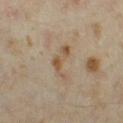notes = catalogued during a skin exam; not biopsied | imaging modality = 15 mm crop, total-body photography | subject = female, in their mid-30s | automated lesion analysis = a footprint of about 5.5 mm² and a symmetry-axis asymmetry near 0.7; a border-irregularity rating of about 8/10 and a color-variation rating of about 3.5/10; an automated nevus-likeness rating near 0 out of 100 | lesion size = ≈4.5 mm | illumination = cross-polarized illumination | site = the right thigh.